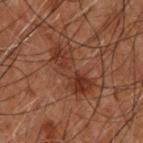The lesion was photographed on a routine skin check and not biopsied; there is no pathology result. A 15 mm crop from a total-body photograph taken for skin-cancer surveillance. The tile uses cross-polarized illumination. The recorded lesion diameter is about 6 mm. A male patient, aged around 50. The lesion is located on the upper back.